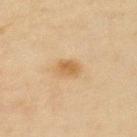{"biopsy_status": "not biopsied; imaged during a skin examination", "lighting": "cross-polarized", "lesion_size": {"long_diameter_mm_approx": 3.0}, "image": {"source": "total-body photography crop", "field_of_view_mm": 15}, "patient": {"sex": "female", "age_approx": 55}, "site": "arm"}